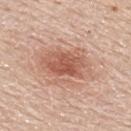Recorded during total-body skin imaging; not selected for excision or biopsy.
The lesion's longest dimension is about 6.5 mm.
Located on the upper back.
Automated tile analysis of the lesion measured a lesion area of about 21 mm², a shape eccentricity near 0.75, and a symmetry-axis asymmetry near 0.2. The software also gave a border-irregularity rating of about 3.5/10 and a within-lesion color-variation index near 5.5/10. And it measured a classifier nevus-likeness of about 80/100 and a lesion-detection confidence of about 100/100.
The tile uses white-light illumination.
Cropped from a whole-body photographic skin survey; the tile spans about 15 mm.
The subject is a male roughly 60 years of age.